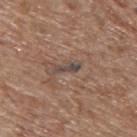Captured during whole-body skin photography for melanoma surveillance; the lesion was not biopsied.
Captured under white-light illumination.
The patient is a male aged 68–72.
This image is a 15 mm lesion crop taken from a total-body photograph.
About 3 mm across.
On the back.
The lesion-visualizer software estimated border irregularity of about 4.5 on a 0–10 scale, internal color variation of about 0 on a 0–10 scale, and a peripheral color-asymmetry measure near 0. The software also gave a classifier nevus-likeness of about 0/100.